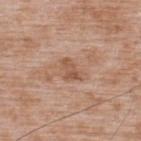The lesion is located on the upper back.
Cropped from a whole-body photographic skin survey; the tile spans about 15 mm.
A male patient aged 48–52.